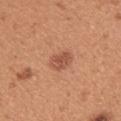Q: Was a biopsy performed?
A: no biopsy performed (imaged during a skin exam)
Q: Who is the patient?
A: female, approximately 45 years of age
Q: How was this image acquired?
A: ~15 mm crop, total-body skin-cancer survey
Q: What is the lesion's diameter?
A: ~2.5 mm (longest diameter)
Q: Where on the body is the lesion?
A: the left upper arm